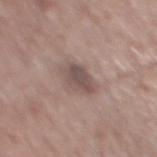A male patient roughly 65 years of age.
The lesion is on the back.
Cropped from a whole-body photographic skin survey; the tile spans about 15 mm.
The tile uses white-light illumination.
The lesion's longest dimension is about 3.5 mm.